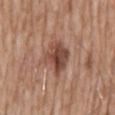Findings:
* follow-up — no biopsy performed (imaged during a skin exam)
* subject — male, approximately 60 years of age
* illumination — white-light
* diameter — about 4.5 mm
* image source — ~15 mm crop, total-body skin-cancer survey
* TBP lesion metrics — a classifier nevus-likeness of about 35/100 and lesion-presence confidence of about 100/100
* body site — the abdomen